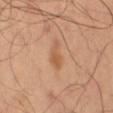Impression: Imaged during a routine full-body skin examination; the lesion was not biopsied and no histopathology is available. Clinical summary: A male patient in their mid- to late 60s. A region of skin cropped from a whole-body photographic capture, roughly 15 mm wide. Automated image analysis of the tile measured an eccentricity of roughly 0.95 and two-axis asymmetry of about 0.45. It also reported a within-lesion color-variation index near 0.5/10 and radial color variation of about 0. The software also gave a nevus-likeness score of about 40/100. Located on the left thigh.